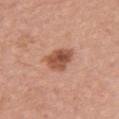anatomic site: the chest | image source: 15 mm crop, total-body photography | tile lighting: white-light illumination | patient: female, aged 58–62 | lesion size: ≈3.5 mm.